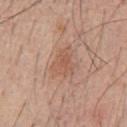Imaged during a routine full-body skin examination; the lesion was not biopsied and no histopathology is available.
The lesion is on the chest.
Automated image analysis of the tile measured a classifier nevus-likeness of about 15/100 and a detector confidence of about 100 out of 100 that the crop contains a lesion.
A male patient aged around 45.
A lesion tile, about 15 mm wide, cut from a 3D total-body photograph.